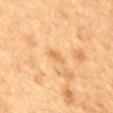biopsy status: total-body-photography surveillance lesion; no biopsy
illumination: cross-polarized illumination
patient: female, aged around 55
location: the mid back
acquisition: total-body-photography crop, ~15 mm field of view
diameter: ~2.5 mm (longest diameter)
image-analysis metrics: a lesion area of about 2 mm², an eccentricity of roughly 0.9, and a symmetry-axis asymmetry near 0.45; a mean CIELAB color near L≈59 a*≈19 b*≈39, a lesion–skin lightness drop of about 7, and a lesion-to-skin contrast of about 5 (normalized; higher = more distinct); a border-irregularity index near 4.5/10, internal color variation of about 0 on a 0–10 scale, and peripheral color asymmetry of about 0